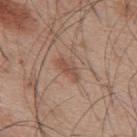biopsy_status: not biopsied; imaged during a skin examination
site: back
patient:
  sex: male
  age_approx: 55
automated_metrics:
  area_mm2_approx: 3.5
  eccentricity: 0.9
  shape_asymmetry: 0.4
  peripheral_color_asymmetry: 0.0
  nevus_likeness_0_100: 25
  lesion_detection_confidence_0_100: 100
image:
  source: total-body photography crop
  field_of_view_mm: 15
lesion_size:
  long_diameter_mm_approx: 3.0
lighting: white-light